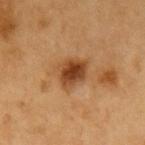The lesion was photographed on a routine skin check and not biopsied; there is no pathology result. From the left upper arm. Approximately 3.5 mm at its widest. A male subject, approximately 60 years of age. Automated tile analysis of the lesion measured a lesion color around L≈44 a*≈24 b*≈38 in CIELAB and roughly 14 lightness units darker than nearby skin. And it measured border irregularity of about 2 on a 0–10 scale, internal color variation of about 5.5 on a 0–10 scale, and radial color variation of about 1.5. This image is a 15 mm lesion crop taken from a total-body photograph. Imaged with cross-polarized lighting.